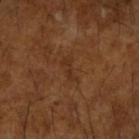{
  "biopsy_status": "not biopsied; imaged during a skin examination",
  "lighting": "cross-polarized",
  "automated_metrics": {
    "area_mm2_approx": 3.0,
    "shape_asymmetry": 0.35,
    "cielab_L": 31,
    "cielab_a": 19,
    "cielab_b": 31,
    "vs_skin_darker_L": 5.0,
    "vs_skin_contrast_norm": 5.0
  },
  "lesion_size": {
    "long_diameter_mm_approx": 3.0
  },
  "patient": {
    "sex": "male",
    "age_approx": 65
  },
  "site": "right upper arm",
  "image": {
    "source": "total-body photography crop",
    "field_of_view_mm": 15
  }
}